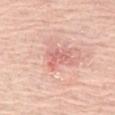  biopsy_status: not biopsied; imaged during a skin examination
  image:
    source: total-body photography crop
    field_of_view_mm: 15
  site: left thigh
  patient:
    sex: male
    age_approx: 80
  automated_metrics:
    border_irregularity_0_10: 6.5
    color_variation_0_10: 0.5
    peripheral_color_asymmetry: 0.0
    nevus_likeness_0_100: 0
    lesion_detection_confidence_0_100: 100
  lesion_size:
    long_diameter_mm_approx: 3.0